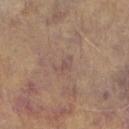{"biopsy_status": "not biopsied; imaged during a skin examination", "image": {"source": "total-body photography crop", "field_of_view_mm": 15}, "site": "arm", "patient": {"sex": "male", "age_approx": 60}, "automated_metrics": {"border_irregularity_0_10": 5.0, "color_variation_0_10": 0.0, "peripheral_color_asymmetry": 0.0, "nevus_likeness_0_100": 0, "lesion_detection_confidence_0_100": 100}, "lighting": "white-light"}